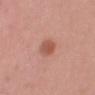This lesion was catalogued during total-body skin photography and was not selected for biopsy.
From the chest.
Automated tile analysis of the lesion measured a nevus-likeness score of about 95/100 and a detector confidence of about 100 out of 100 that the crop contains a lesion.
The subject is a female roughly 40 years of age.
A roughly 15 mm field-of-view crop from a total-body skin photograph.
Captured under white-light illumination.
Longest diameter approximately 2.5 mm.